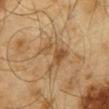Recorded during total-body skin imaging; not selected for excision or biopsy. On the mid back. The tile uses cross-polarized illumination. A 15 mm close-up tile from a total-body photography series done for melanoma screening. The subject is a male aged approximately 65. Approximately 4.5 mm at its widest.